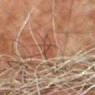Clinical impression: Recorded during total-body skin imaging; not selected for excision or biopsy. Clinical summary: Located on the left thigh. A male subject aged 78–82. A close-up tile cropped from a whole-body skin photograph, about 15 mm across. Imaged with cross-polarized lighting.